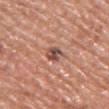This lesion was catalogued during total-body skin photography and was not selected for biopsy.
The lesion is located on the front of the torso.
A female subject, about 60 years old.
Cropped from a whole-body photographic skin survey; the tile spans about 15 mm.